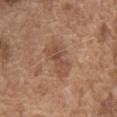workup: imaged on a skin check; not biopsied | size: ~5.5 mm (longest diameter) | image source: 15 mm crop, total-body photography | automated metrics: an area of roughly 10 mm², a shape eccentricity near 0.9, and two-axis asymmetry of about 0.3; a normalized lesion–skin contrast near 6; a classifier nevus-likeness of about 5/100 and a lesion-detection confidence of about 100/100 | subject: male, roughly 75 years of age | illumination: white-light illumination | anatomic site: the chest.